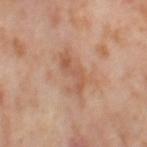Recorded during total-body skin imaging; not selected for excision or biopsy.
A female subject about 55 years old.
Approximately 5 mm at its widest.
A roughly 15 mm field-of-view crop from a total-body skin photograph.
The total-body-photography lesion software estimated an area of roughly 8.5 mm², a shape eccentricity near 0.85, and a shape-asymmetry score of about 0.4 (0 = symmetric). And it measured a lesion–skin lightness drop of about 8 and a lesion-to-skin contrast of about 5.5 (normalized; higher = more distinct). And it measured a border-irregularity index near 6/10 and a within-lesion color-variation index near 3/10. The analysis additionally found a lesion-detection confidence of about 100/100.
The lesion is located on the right thigh.